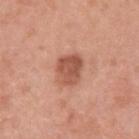Clinical impression: The lesion was photographed on a routine skin check and not biopsied; there is no pathology result. Image and clinical context: A female patient aged around 40. Cropped from a total-body skin-imaging series; the visible field is about 15 mm. The recorded lesion diameter is about 4 mm. The lesion is located on the left upper arm. Imaged with white-light lighting.